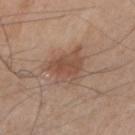Captured during whole-body skin photography for melanoma surveillance; the lesion was not biopsied. The lesion is located on the right upper arm. A male subject aged 53 to 57. This image is a 15 mm lesion crop taken from a total-body photograph. The lesion's longest dimension is about 5 mm. Imaged with white-light lighting.